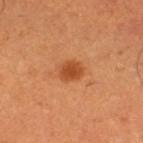biopsy_status: not biopsied; imaged during a skin examination
site: left thigh
image:
  source: total-body photography crop
  field_of_view_mm: 15
patient:
  sex: male
  age_approx: 50